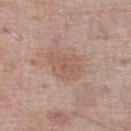Automated tile analysis of the lesion measured a footprint of about 11 mm² and a symmetry-axis asymmetry near 0.25. It also reported about 7 CIELAB-L* units darker than the surrounding skin and a lesion-to-skin contrast of about 5 (normalized; higher = more distinct). And it measured border irregularity of about 2.5 on a 0–10 scale and internal color variation of about 2 on a 0–10 scale. And it measured a detector confidence of about 100 out of 100 that the crop contains a lesion.
Located on the right lower leg.
A 15 mm close-up extracted from a 3D total-body photography capture.
About 5 mm across.
A male subject about 70 years old.
This is a white-light tile.